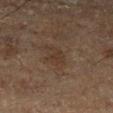biopsy_status: not biopsied; imaged during a skin examination
image:
  source: total-body photography crop
  field_of_view_mm: 15
lighting: cross-polarized
lesion_size:
  long_diameter_mm_approx: 2.5
site: left lower leg
patient:
  sex: male
  age_approx: 75